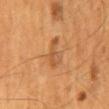notes = no biopsy performed (imaged during a skin exam)
illumination = cross-polarized
patient = male, roughly 55 years of age
automated lesion analysis = an automated nevus-likeness rating near 0 out of 100
lesion size = about 4 mm
acquisition = total-body-photography crop, ~15 mm field of view
location = the back